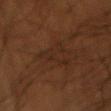{
  "patient": {
    "sex": "male",
    "age_approx": 50
  },
  "image": {
    "source": "total-body photography crop",
    "field_of_view_mm": 15
  },
  "site": "left upper arm"
}